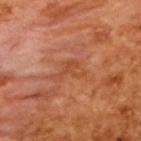follow-up: total-body-photography surveillance lesion; no biopsy | size: about 4 mm | image source: ~15 mm tile from a whole-body skin photo | patient: male, in their mid- to late 60s | automated metrics: a peripheral color-asymmetry measure near 1; a detector confidence of about 95 out of 100 that the crop contains a lesion.